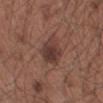Captured during whole-body skin photography for melanoma surveillance; the lesion was not biopsied. The lesion is located on the left upper arm. A male subject in their mid-50s. Automated tile analysis of the lesion measured a lesion color around L≈37 a*≈19 b*≈22 in CIELAB, a lesion–skin lightness drop of about 10, and a normalized border contrast of about 8.5. And it measured a detector confidence of about 100 out of 100 that the crop contains a lesion. A 15 mm close-up extracted from a 3D total-body photography capture. Imaged with white-light lighting.